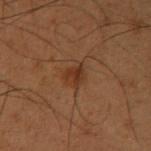Impression:
The lesion was tiled from a total-body skin photograph and was not biopsied.
Clinical summary:
The lesion's longest dimension is about 2.5 mm. A male patient, roughly 55 years of age. Cropped from a whole-body photographic skin survey; the tile spans about 15 mm. The lesion is located on the right upper arm. Imaged with cross-polarized lighting. Automated tile analysis of the lesion measured a mean CIELAB color near L≈27 a*≈17 b*≈26, a lesion–skin lightness drop of about 6, and a normalized border contrast of about 7. It also reported a detector confidence of about 100 out of 100 that the crop contains a lesion.